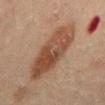Assessment: The lesion was tiled from a total-body skin photograph and was not biopsied. Clinical summary: A male patient roughly 45 years of age. Captured under cross-polarized illumination. From the abdomen. Cropped from a whole-body photographic skin survey; the tile spans about 15 mm.